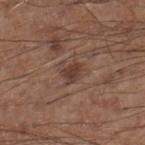biopsy status: no biopsy performed (imaged during a skin exam)
site: the right lower leg
image source: ~15 mm crop, total-body skin-cancer survey
image-analysis metrics: a border-irregularity rating of about 3.5/10, a within-lesion color-variation index near 2/10, and a peripheral color-asymmetry measure near 1; an automated nevus-likeness rating near 65 out of 100 and lesion-presence confidence of about 100/100
subject: male, approximately 60 years of age
lesion size: about 2.5 mm
illumination: white-light illumination